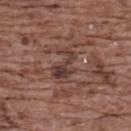notes — imaged on a skin check; not biopsied
acquisition — ~15 mm tile from a whole-body skin photo
patient — female, aged around 75
location — the upper back
tile lighting — white-light
image-analysis metrics — a lesion color around L≈38 a*≈19 b*≈22 in CIELAB, about 9 CIELAB-L* units darker than the surrounding skin, and a normalized lesion–skin contrast near 7.5; a classifier nevus-likeness of about 0/100 and a detector confidence of about 80 out of 100 that the crop contains a lesion